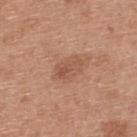Recorded during total-body skin imaging; not selected for excision or biopsy.